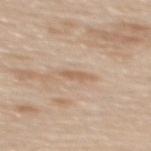Clinical impression:
Imaged during a routine full-body skin examination; the lesion was not biopsied and no histopathology is available.
Context:
Automated tile analysis of the lesion measured a footprint of about 2.5 mm², an outline eccentricity of about 0.9 (0 = round, 1 = elongated), and a symmetry-axis asymmetry near 0.35. And it measured roughly 8 lightness units darker than nearby skin and a normalized lesion–skin contrast near 6. A female patient, aged around 60. Measured at roughly 2.5 mm in maximum diameter. The lesion is located on the upper back. Cropped from a total-body skin-imaging series; the visible field is about 15 mm.